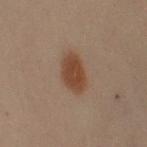Captured during whole-body skin photography for melanoma surveillance; the lesion was not biopsied.
A male subject aged approximately 50.
A 15 mm close-up tile from a total-body photography series done for melanoma screening.
The lesion is on the left arm.
Automated tile analysis of the lesion measured an area of roughly 9.5 mm², an eccentricity of roughly 0.8, and two-axis asymmetry of about 0.2. The analysis additionally found a border-irregularity index near 2/10 and a color-variation rating of about 2.5/10. It also reported a lesion-detection confidence of about 100/100.
The lesion's longest dimension is about 4.5 mm.
The tile uses cross-polarized illumination.